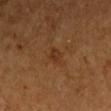follow-up = catalogued during a skin exam; not biopsied
location = the right upper arm
patient = male, approximately 55 years of age
lighting = cross-polarized illumination
image-analysis metrics = a lesion area of about 3.5 mm², an eccentricity of roughly 0.8, and a symmetry-axis asymmetry near 0.2; a mean CIELAB color near L≈30 a*≈19 b*≈30, a lesion–skin lightness drop of about 6, and a normalized lesion–skin contrast near 6; border irregularity of about 2.5 on a 0–10 scale; a nevus-likeness score of about 0/100 and a detector confidence of about 100 out of 100 that the crop contains a lesion
imaging modality = 15 mm crop, total-body photography